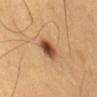<lesion>
<biopsy_status>not biopsied; imaged during a skin examination</biopsy_status>
<patient>
  <sex>male</sex>
  <age_approx>60</age_approx>
</patient>
<image>
  <source>total-body photography crop</source>
  <field_of_view_mm>15</field_of_view_mm>
</image>
<lesion_size>
  <long_diameter_mm_approx>3.0</long_diameter_mm_approx>
</lesion_size>
<lighting>cross-polarized</lighting>
<site>lower back</site>
</lesion>